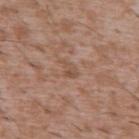The lesion was photographed on a routine skin check and not biopsied; there is no pathology result.